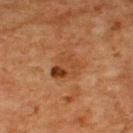Imaged during a routine full-body skin examination; the lesion was not biopsied and no histopathology is available. The recorded lesion diameter is about 4 mm. A male subject about 65 years old. Cropped from a total-body skin-imaging series; the visible field is about 15 mm. The lesion is on the upper back. Automated tile analysis of the lesion measured a classifier nevus-likeness of about 5/100 and a lesion-detection confidence of about 100/100.